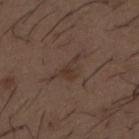| field | value |
|---|---|
| notes | catalogued during a skin exam; not biopsied |
| automated lesion analysis | a lesion area of about 3.5 mm², an eccentricity of roughly 0.85, and two-axis asymmetry of about 0.6; a color-variation rating of about 1.5/10 |
| imaging modality | 15 mm crop, total-body photography |
| lighting | white-light |
| patient | male, aged 48–52 |
| location | the mid back |